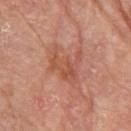Imaged during a routine full-body skin examination; the lesion was not biopsied and no histopathology is available.
A male subject aged around 80.
An algorithmic analysis of the crop reported border irregularity of about 8 on a 0–10 scale, a within-lesion color-variation index near 3.5/10, and peripheral color asymmetry of about 1. And it measured a classifier nevus-likeness of about 0/100 and lesion-presence confidence of about 100/100.
The lesion's longest dimension is about 4.5 mm.
Located on the right upper arm.
A close-up tile cropped from a whole-body skin photograph, about 15 mm across.